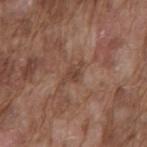automated metrics — an area of roughly 3.5 mm², a shape eccentricity near 0.8, and a shape-asymmetry score of about 0.3 (0 = symmetric); internal color variation of about 2.5 on a 0–10 scale and radial color variation of about 1; a nevus-likeness score of about 0/100 and a detector confidence of about 95 out of 100 that the crop contains a lesion
image — ~15 mm tile from a whole-body skin photo
patient — male, about 75 years old
size — ~3 mm (longest diameter)
illumination — white-light illumination
site — the mid back